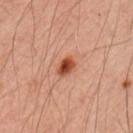biopsy status — no biopsy performed (imaged during a skin exam); acquisition — ~15 mm crop, total-body skin-cancer survey; patient — male, approximately 45 years of age; lesion size — ≈2.5 mm; site — the left upper arm; lighting — cross-polarized illumination.